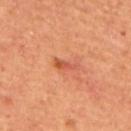follow-up — imaged on a skin check; not biopsied
subject — male, in their mid- to late 60s
illumination — cross-polarized
body site — the back
image — ~15 mm tile from a whole-body skin photo